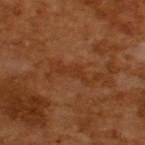Recorded during total-body skin imaging; not selected for excision or biopsy. A male subject aged 63 to 67. A 15 mm close-up tile from a total-body photography series done for melanoma screening. Automated tile analysis of the lesion measured a lesion area of about 2.5 mm², an eccentricity of roughly 0.95, and two-axis asymmetry of about 0.7. The tile uses cross-polarized illumination.